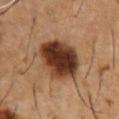Findings:
• notes — imaged on a skin check; not biopsied
• automated metrics — a lesion area of about 22 mm² and an outline eccentricity of about 0.55 (0 = round, 1 = elongated); border irregularity of about 1.5 on a 0–10 scale, internal color variation of about 8.5 on a 0–10 scale, and peripheral color asymmetry of about 2.5
• subject — male, in their mid-50s
• image — 15 mm crop, total-body photography
• illumination — cross-polarized
• anatomic site — the chest
• diameter — about 5.5 mm